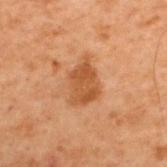  biopsy_status: not biopsied; imaged during a skin examination
  lighting: cross-polarized
  site: upper back
  image:
    source: total-body photography crop
    field_of_view_mm: 15
  lesion_size:
    long_diameter_mm_approx: 4.5
  patient:
    sex: male
    age_approx: 70